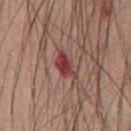Clinical impression:
Captured during whole-body skin photography for melanoma surveillance; the lesion was not biopsied.
Image and clinical context:
Longest diameter approximately 4 mm. The lesion-visualizer software estimated an average lesion color of about L≈41 a*≈29 b*≈23 (CIELAB), roughly 12 lightness units darker than nearby skin, and a lesion-to-skin contrast of about 9.5 (normalized; higher = more distinct). The analysis additionally found lesion-presence confidence of about 100/100. A roughly 15 mm field-of-view crop from a total-body skin photograph. Located on the chest. This is a white-light tile. A male subject approximately 60 years of age.